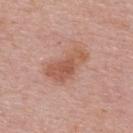The lesion was tiled from a total-body skin photograph and was not biopsied.
The lesion's longest dimension is about 5.5 mm.
The lesion is located on the upper back.
The subject is a female aged around 50.
A lesion tile, about 15 mm wide, cut from a 3D total-body photograph.